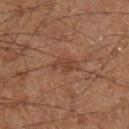No biopsy was performed on this lesion — it was imaged during a full skin examination and was not determined to be concerning.